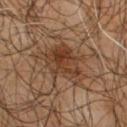Recorded during total-body skin imaging; not selected for excision or biopsy.
The patient is a male roughly 65 years of age.
A 15 mm crop from a total-body photograph taken for skin-cancer surveillance.
Longest diameter approximately 5.5 mm.
Imaged with cross-polarized lighting.
From the back.